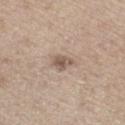Q: Was this lesion biopsied?
A: imaged on a skin check; not biopsied
Q: How was the tile lit?
A: white-light
Q: Patient demographics?
A: male, approximately 70 years of age
Q: Automated lesion metrics?
A: a color-variation rating of about 3.5/10 and radial color variation of about 1.5; an automated nevus-likeness rating near 35 out of 100 and a lesion-detection confidence of about 100/100
Q: How was this image acquired?
A: total-body-photography crop, ~15 mm field of view
Q: Lesion location?
A: the left lower leg